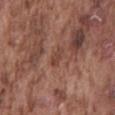Recorded during total-body skin imaging; not selected for excision or biopsy. The patient is a male aged 73 to 77. The lesion is on the back. The recorded lesion diameter is about 3 mm. Imaged with white-light lighting. This image is a 15 mm lesion crop taken from a total-body photograph. An algorithmic analysis of the crop reported an eccentricity of roughly 0.9 and a symmetry-axis asymmetry near 0.3. And it measured a lesion color around L≈43 a*≈22 b*≈26 in CIELAB and a normalized lesion–skin contrast near 5.5. And it measured border irregularity of about 3 on a 0–10 scale, a within-lesion color-variation index near 0.5/10, and peripheral color asymmetry of about 0.